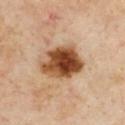<case>
  <site>chest</site>
  <lesion_size>
    <long_diameter_mm_approx>5.0</long_diameter_mm_approx>
  </lesion_size>
  <patient>
    <sex>male</sex>
    <age_approx>55</age_approx>
  </patient>
  <image>
    <source>total-body photography crop</source>
    <field_of_view_mm>15</field_of_view_mm>
  </image>
</case>